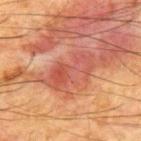notes: total-body-photography surveillance lesion; no biopsy
diameter: ~6 mm (longest diameter)
lighting: cross-polarized
acquisition: 15 mm crop, total-body photography
body site: the upper back
subject: male, about 65 years old